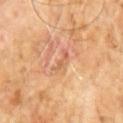No biopsy was performed on this lesion — it was imaged during a full skin examination and was not determined to be concerning.
Located on the abdomen.
A region of skin cropped from a whole-body photographic capture, roughly 15 mm wide.
Measured at roughly 3.5 mm in maximum diameter.
A male subject roughly 60 years of age.
The tile uses cross-polarized illumination.
The lesion-visualizer software estimated a mean CIELAB color near L≈59 a*≈24 b*≈34, roughly 7 lightness units darker than nearby skin, and a normalized lesion–skin contrast near 4.5.